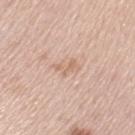lighting: white-light; subject: female, about 60 years old; automated lesion analysis: a lesion area of about 3.5 mm², an eccentricity of roughly 0.8, and two-axis asymmetry of about 0.5; location: the arm; lesion size: ~2.5 mm (longest diameter); image source: ~15 mm crop, total-body skin-cancer survey.